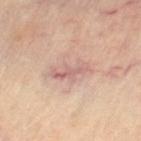The lesion was photographed on a routine skin check and not biopsied; there is no pathology result.